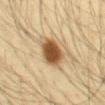The lesion is on the abdomen.
This is a cross-polarized tile.
A roughly 15 mm field-of-view crop from a total-body skin photograph.
Approximately 4 mm at its widest.
A male subject aged around 35.
An algorithmic analysis of the crop reported a normalized lesion–skin contrast near 12. It also reported a border-irregularity index near 1/10, a color-variation rating of about 4/10, and radial color variation of about 1.